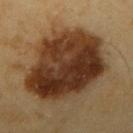workup=imaged on a skin check; not biopsied